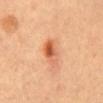From the mid back.
A 15 mm crop from a total-body photograph taken for skin-cancer surveillance.
The patient is a female roughly 60 years of age.
Longest diameter approximately 3.5 mm.
Automated tile analysis of the lesion measured an area of roughly 5.5 mm², an eccentricity of roughly 0.85, and a symmetry-axis asymmetry near 0.15. It also reported a border-irregularity rating of about 2/10 and peripheral color asymmetry of about 3. The analysis additionally found an automated nevus-likeness rating near 90 out of 100 and a detector confidence of about 100 out of 100 that the crop contains a lesion.
Captured under cross-polarized illumination.